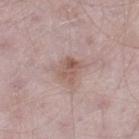| field | value |
|---|---|
| biopsy status | catalogued during a skin exam; not biopsied |
| acquisition | ~15 mm tile from a whole-body skin photo |
| TBP lesion metrics | a lesion area of about 5.5 mm² and a symmetry-axis asymmetry near 0.25; roughly 9 lightness units darker than nearby skin and a normalized border contrast of about 7; border irregularity of about 2.5 on a 0–10 scale and a color-variation rating of about 5/10; lesion-presence confidence of about 100/100 |
| site | the leg |
| size | about 3 mm |
| subject | male, aged around 50 |
| lighting | white-light illumination |